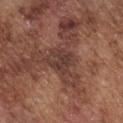Findings:
- subject — male, aged 73–77
- site — the chest
- image — total-body-photography crop, ~15 mm field of view